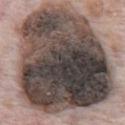Clinical impression:
Captured during whole-body skin photography for melanoma surveillance; the lesion was not biopsied.
Acquisition and patient details:
Approximately 14.5 mm at its widest. The lesion is on the front of the torso. A roughly 15 mm field-of-view crop from a total-body skin photograph. Captured under white-light illumination. A male patient aged 68 to 72. The total-body-photography lesion software estimated an average lesion color of about L≈42 a*≈10 b*≈16 (CIELAB), roughly 23 lightness units darker than nearby skin, and a normalized lesion–skin contrast near 16.5.